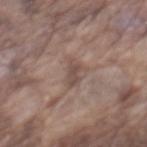Assessment:
Imaged during a routine full-body skin examination; the lesion was not biopsied and no histopathology is available.
Background:
From the mid back. The lesion-visualizer software estimated a lesion area of about 3.5 mm², an eccentricity of roughly 0.95, and a symmetry-axis asymmetry near 0.4. The analysis additionally found an average lesion color of about L≈48 a*≈15 b*≈22 (CIELAB) and a normalized border contrast of about 6.5. And it measured a border-irregularity rating of about 4.5/10, internal color variation of about 2.5 on a 0–10 scale, and radial color variation of about 1. Approximately 3.5 mm at its widest. Captured under white-light illumination. A male subject, in their mid-70s. This image is a 15 mm lesion crop taken from a total-body photograph.